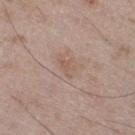Imaged during a routine full-body skin examination; the lesion was not biopsied and no histopathology is available.
A lesion tile, about 15 mm wide, cut from a 3D total-body photograph.
On the leg.
The patient is a male aged approximately 50.